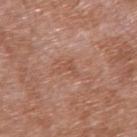site: right upper arm
patient:
  sex: male
  age_approx: 60
image:
  source: total-body photography crop
  field_of_view_mm: 15